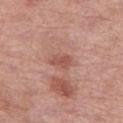The lesion is on the right thigh. A female patient, roughly 55 years of age. A roughly 15 mm field-of-view crop from a total-body skin photograph. Automated image analysis of the tile measured a footprint of about 3.5 mm² and a symmetry-axis asymmetry near 0.3. The software also gave a mean CIELAB color near L≈52 a*≈26 b*≈27 and a normalized lesion–skin contrast near 6. The analysis additionally found a border-irregularity index near 3/10, a color-variation rating of about 1.5/10, and peripheral color asymmetry of about 0.5. It also reported a classifier nevus-likeness of about 0/100 and a detector confidence of about 100 out of 100 that the crop contains a lesion.